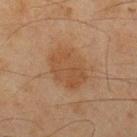  biopsy_status: not biopsied; imaged during a skin examination
  lesion_size:
    long_diameter_mm_approx: 5.5
  image:
    source: total-body photography crop
    field_of_view_mm: 15
  automated_metrics:
    area_mm2_approx: 17.0
    border_irregularity_0_10: 2.5
    color_variation_0_10: 2.5
    peripheral_color_asymmetry: 1.0
    nevus_likeness_0_100: 40
    lesion_detection_confidence_0_100: 100
  patient:
    sex: male
    age_approx: 45
  site: upper back
  lighting: cross-polarized